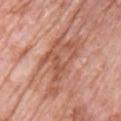Impression:
Recorded during total-body skin imaging; not selected for excision or biopsy.
Background:
Located on the chest. Captured under white-light illumination. About 7.5 mm across. A region of skin cropped from a whole-body photographic capture, roughly 15 mm wide. A male patient, aged 78 to 82.